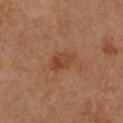Findings:
- subject: female, aged approximately 45
- automated lesion analysis: two-axis asymmetry of about 0.2; a lesion color around L≈36 a*≈21 b*≈30 in CIELAB, a lesion–skin lightness drop of about 6, and a lesion-to-skin contrast of about 6.5 (normalized; higher = more distinct); a border-irregularity rating of about 2/10 and a within-lesion color-variation index near 4/10
- lesion diameter: ~3.5 mm (longest diameter)
- acquisition: 15 mm crop, total-body photography
- site: the chest